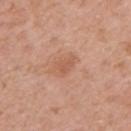Imaged during a routine full-body skin examination; the lesion was not biopsied and no histopathology is available.
The lesion's longest dimension is about 2.5 mm.
A region of skin cropped from a whole-body photographic capture, roughly 15 mm wide.
The lesion is located on the right upper arm.
The subject is a female aged around 45.
An algorithmic analysis of the crop reported a lesion area of about 3.5 mm² and an outline eccentricity of about 0.7 (0 = round, 1 = elongated). The software also gave a normalized border contrast of about 5. It also reported border irregularity of about 3 on a 0–10 scale, a within-lesion color-variation index near 1.5/10, and peripheral color asymmetry of about 0.5.